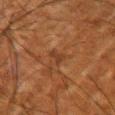| feature | finding |
|---|---|
| biopsy status | total-body-photography surveillance lesion; no biopsy |
| site | the right upper arm |
| patient | male, about 65 years old |
| acquisition | 15 mm crop, total-body photography |